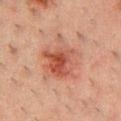Q: Was this lesion biopsied?
A: no biopsy performed (imaged during a skin exam)
Q: Patient demographics?
A: male, aged around 50
Q: What kind of image is this?
A: 15 mm crop, total-body photography
Q: Where on the body is the lesion?
A: the chest